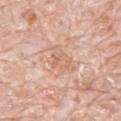The lesion was photographed on a routine skin check and not biopsied; there is no pathology result.
The lesion is located on the mid back.
The subject is a male aged around 80.
A close-up tile cropped from a whole-body skin photograph, about 15 mm across.